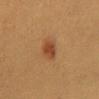Notes:
- notes — total-body-photography surveillance lesion; no biopsy
- size — ~3 mm (longest diameter)
- acquisition — ~15 mm tile from a whole-body skin photo
- location — the chest
- patient — female, aged 38–42